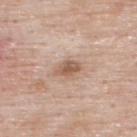Notes:
- subject — male, aged approximately 55
- image-analysis metrics — an area of roughly 4.5 mm², a shape eccentricity near 0.75, and a shape-asymmetry score of about 0.2 (0 = symmetric); an average lesion color of about L≈57 a*≈19 b*≈29 (CIELAB) and a lesion-to-skin contrast of about 8 (normalized; higher = more distinct)
- lesion size — ≈3 mm
- location — the upper back
- image source — 15 mm crop, total-body photography